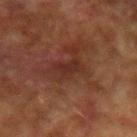Recorded during total-body skin imaging; not selected for excision or biopsy.
The lesion-visualizer software estimated lesion-presence confidence of about 100/100.
The patient is a male in their mid-70s.
The lesion is on the left forearm.
Cropped from a whole-body photographic skin survey; the tile spans about 15 mm.
Measured at roughly 3 mm in maximum diameter.
Imaged with cross-polarized lighting.